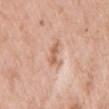Impression:
Part of a total-body skin-imaging series; this lesion was reviewed on a skin check and was not flagged for biopsy.
Background:
Imaged with white-light lighting. A roughly 15 mm field-of-view crop from a total-body skin photograph. The patient is a female aged 63 to 67. Approximately 3 mm at its widest. On the right upper arm.